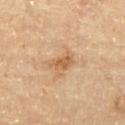Clinical impression: This lesion was catalogued during total-body skin photography and was not selected for biopsy.